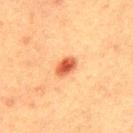Part of a total-body skin-imaging series; this lesion was reviewed on a skin check and was not flagged for biopsy.
This is a cross-polarized tile.
Measured at roughly 3 mm in maximum diameter.
A 15 mm close-up tile from a total-body photography series done for melanoma screening.
A male subject, approximately 40 years of age.
The lesion is located on the mid back.
The total-body-photography lesion software estimated internal color variation of about 3 on a 0–10 scale. And it measured an automated nevus-likeness rating near 100 out of 100 and a detector confidence of about 100 out of 100 that the crop contains a lesion.